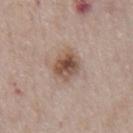Recorded during total-body skin imaging; not selected for excision or biopsy.
The patient is a male aged 73–77.
The lesion is on the front of the torso.
Longest diameter approximately 4 mm.
A 15 mm close-up tile from a total-body photography series done for melanoma screening.
Captured under white-light illumination.